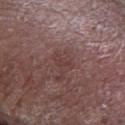biopsy status: imaged on a skin check; not biopsied
patient: male, aged 73–77
image: ~15 mm tile from a whole-body skin photo
anatomic site: the left lower leg